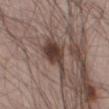follow-up = total-body-photography surveillance lesion; no biopsy | image source = total-body-photography crop, ~15 mm field of view | body site = the left thigh | subject = male, aged 53–57.